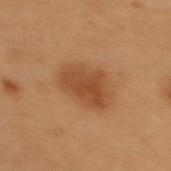Recorded during total-body skin imaging; not selected for excision or biopsy.
From the upper back.
Imaged with cross-polarized lighting.
Longest diameter approximately 5 mm.
Cropped from a total-body skin-imaging series; the visible field is about 15 mm.
The patient is a female aged around 50.